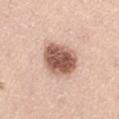Notes:
• biopsy status — total-body-photography surveillance lesion; no biopsy
• site — the left thigh
• acquisition — ~15 mm tile from a whole-body skin photo
• automated lesion analysis — a classifier nevus-likeness of about 75/100
• lesion size — about 4.5 mm
• tile lighting — white-light illumination
• subject — male, aged approximately 65